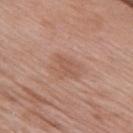Notes:
- biopsy status: catalogued during a skin exam; not biopsied
- patient: female, aged 68–72
- site: the chest
- tile lighting: white-light
- image source: ~15 mm crop, total-body skin-cancer survey